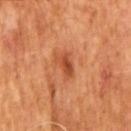The lesion was tiled from a total-body skin photograph and was not biopsied.
The subject is a male approximately 65 years of age.
The lesion-visualizer software estimated an area of roughly 4 mm² and an outline eccentricity of about 0.85 (0 = round, 1 = elongated). It also reported a border-irregularity index near 3.5/10, a within-lesion color-variation index near 2/10, and peripheral color asymmetry of about 0.5.
The tile uses cross-polarized illumination.
A 15 mm crop from a total-body photograph taken for skin-cancer surveillance.
The lesion's longest dimension is about 3 mm.